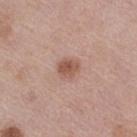Q: Is there a histopathology result?
A: catalogued during a skin exam; not biopsied
Q: Where on the body is the lesion?
A: the right thigh
Q: Who is the patient?
A: female, approximately 40 years of age
Q: What did automated image analysis measure?
A: an area of roughly 5.5 mm², an eccentricity of roughly 0.55, and two-axis asymmetry of about 0.2; an average lesion color of about L≈54 a*≈21 b*≈27 (CIELAB), a lesion–skin lightness drop of about 10, and a lesion-to-skin contrast of about 7.5 (normalized; higher = more distinct); border irregularity of about 2 on a 0–10 scale and radial color variation of about 1; a classifier nevus-likeness of about 80/100 and a lesion-detection confidence of about 100/100
Q: Illumination type?
A: white-light illumination
Q: How was this image acquired?
A: ~15 mm crop, total-body skin-cancer survey